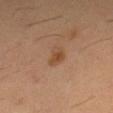Clinical impression: The lesion was tiled from a total-body skin photograph and was not biopsied. Context: A close-up tile cropped from a whole-body skin photograph, about 15 mm across. A male subject aged 58–62. The lesion's longest dimension is about 3 mm. From the right thigh. Imaged with cross-polarized lighting.